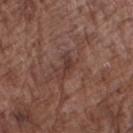body site: the right forearm
imaging modality: ~15 mm tile from a whole-body skin photo
illumination: white-light
patient: male, aged 73 to 77
lesion diameter: ≈3 mm
automated lesion analysis: an area of roughly 3 mm², an eccentricity of roughly 0.85, and a symmetry-axis asymmetry near 0.6; a classifier nevus-likeness of about 0/100 and lesion-presence confidence of about 90/100